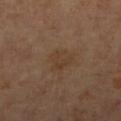{
  "biopsy_status": "not biopsied; imaged during a skin examination",
  "site": "right lower leg",
  "lighting": "cross-polarized",
  "lesion_size": {
    "long_diameter_mm_approx": 3.0
  },
  "patient": {
    "sex": "female",
    "age_approx": 70
  },
  "image": {
    "source": "total-body photography crop",
    "field_of_view_mm": 15
  }
}